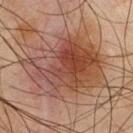Assessment: Imaged during a routine full-body skin examination; the lesion was not biopsied and no histopathology is available.